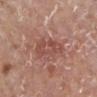Q: Was a biopsy performed?
A: imaged on a skin check; not biopsied